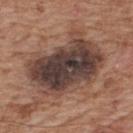{"biopsy_status": "not biopsied; imaged during a skin examination", "image": {"source": "total-body photography crop", "field_of_view_mm": 15}, "lesion_size": {"long_diameter_mm_approx": 8.5}, "automated_metrics": {"cielab_L": 40, "cielab_a": 16, "cielab_b": 21, "vs_skin_darker_L": 15.0, "vs_skin_contrast_norm": 12.5, "color_variation_0_10": 9.5, "peripheral_color_asymmetry": 3.0, "nevus_likeness_0_100": 0}, "lighting": "white-light", "site": "back", "patient": {"sex": "male", "age_approx": 65}}